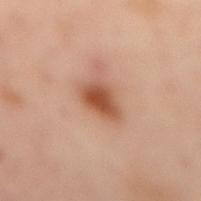{"automated_metrics": {"eccentricity": 0.8, "shape_asymmetry": 0.3, "cielab_L": 43, "cielab_a": 21, "cielab_b": 28, "vs_skin_darker_L": 11.0, "vs_skin_contrast_norm": 9.5}, "image": {"source": "total-body photography crop", "field_of_view_mm": 15}, "site": "back", "lesion_size": {"long_diameter_mm_approx": 4.0}, "patient": {"sex": "female", "age_approx": 55}, "lighting": "cross-polarized"}